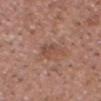<case>
<biopsy_status>not biopsied; imaged during a skin examination</biopsy_status>
<image>
  <source>total-body photography crop</source>
  <field_of_view_mm>15</field_of_view_mm>
</image>
<site>head or neck</site>
<lighting>white-light</lighting>
<patient>
  <sex>male</sex>
  <age_approx>55</age_approx>
</patient>
<lesion_size>
  <long_diameter_mm_approx>3.0</long_diameter_mm_approx>
</lesion_size>
</case>